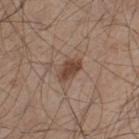biopsy status = catalogued during a skin exam; not biopsied | image source = 15 mm crop, total-body photography | subject = male, approximately 45 years of age | image-analysis metrics = border irregularity of about 2.5 on a 0–10 scale, a color-variation rating of about 2/10, and radial color variation of about 0.5; a nevus-likeness score of about 85/100 and a lesion-detection confidence of about 100/100 | lesion diameter = ~3 mm (longest diameter) | illumination = white-light illumination | location = the left thigh.